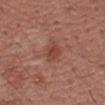Case summary:
- biopsy status: imaged on a skin check; not biopsied
- tile lighting: white-light
- image: ~15 mm crop, total-body skin-cancer survey
- location: the right upper arm
- image-analysis metrics: a footprint of about 4.5 mm², an eccentricity of roughly 0.7, and two-axis asymmetry of about 0.2; a mean CIELAB color near L≈44 a*≈25 b*≈27, a lesion–skin lightness drop of about 8, and a lesion-to-skin contrast of about 6.5 (normalized; higher = more distinct); a border-irregularity index near 2/10, a color-variation rating of about 4/10, and a peripheral color-asymmetry measure near 1.5; a classifier nevus-likeness of about 10/100 and a lesion-detection confidence of about 100/100
- diameter: ~2.5 mm (longest diameter)
- patient: female, aged approximately 55